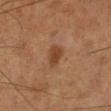Q: Is there a histopathology result?
A: catalogued during a skin exam; not biopsied
Q: Lesion location?
A: the left lower leg
Q: How was this image acquired?
A: ~15 mm tile from a whole-body skin photo
Q: What lighting was used for the tile?
A: cross-polarized
Q: Patient demographics?
A: male, aged around 55
Q: What is the lesion's diameter?
A: ~3 mm (longest diameter)
Q: Automated lesion metrics?
A: a lesion area of about 5 mm², a shape eccentricity near 0.7, and two-axis asymmetry of about 0.25; a classifier nevus-likeness of about 90/100